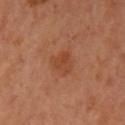Q: Is there a histopathology result?
A: no biopsy performed (imaged during a skin exam)